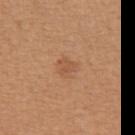No biopsy was performed on this lesion — it was imaged during a full skin examination and was not determined to be concerning. The tile uses white-light illumination. A roughly 15 mm field-of-view crop from a total-body skin photograph. Located on the abdomen. Approximately 2.5 mm at its widest. A male subject, aged around 55.